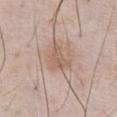<lesion>
  <biopsy_status>not biopsied; imaged during a skin examination</biopsy_status>
  <automated_metrics>
    <vs_skin_darker_L>8.0</vs_skin_darker_L>
    <vs_skin_contrast_norm>6.0</vs_skin_contrast_norm>
    <border_irregularity_0_10>5.0</border_irregularity_0_10>
    <color_variation_0_10>2.5</color_variation_0_10>
    <peripheral_color_asymmetry>1.0</peripheral_color_asymmetry>
    <nevus_likeness_0_100>10</nevus_likeness_0_100>
    <lesion_detection_confidence_0_100>100</lesion_detection_confidence_0_100>
  </automated_metrics>
  <site>chest</site>
  <patient>
    <sex>male</sex>
    <age_approx>55</age_approx>
  </patient>
  <lesion_size>
    <long_diameter_mm_approx>4.0</long_diameter_mm_approx>
  </lesion_size>
  <lighting>white-light</lighting>
  <image>
    <source>total-body photography crop</source>
    <field_of_view_mm>15</field_of_view_mm>
  </image>
</lesion>